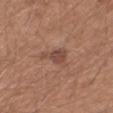On the arm. A lesion tile, about 15 mm wide, cut from a 3D total-body photograph. A male patient, about 50 years old.